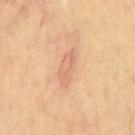Impression: The lesion was tiled from a total-body skin photograph and was not biopsied. Image and clinical context: Approximately 4.5 mm at its widest. A male subject, aged approximately 70. The total-body-photography lesion software estimated a lesion area of about 7 mm², an outline eccentricity of about 0.9 (0 = round, 1 = elongated), and a symmetry-axis asymmetry near 0.2. And it measured a border-irregularity index near 2.5/10, internal color variation of about 3 on a 0–10 scale, and radial color variation of about 1. And it measured lesion-presence confidence of about 100/100. A 15 mm close-up tile from a total-body photography series done for melanoma screening. Imaged with cross-polarized lighting. On the chest.